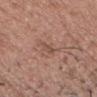| field | value |
|---|---|
| follow-up | catalogued during a skin exam; not biopsied |
| anatomic site | the head or neck |
| image-analysis metrics | a lesion color around L≈50 a*≈20 b*≈26 in CIELAB, a lesion–skin lightness drop of about 7, and a normalized lesion–skin contrast near 5; a border-irregularity rating of about 3.5/10, internal color variation of about 1 on a 0–10 scale, and radial color variation of about 0; a lesion-detection confidence of about 95/100 |
| acquisition | total-body-photography crop, ~15 mm field of view |
| diameter | ~2.5 mm (longest diameter) |
| subject | male, approximately 50 years of age |